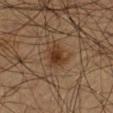Clinical impression:
The lesion was photographed on a routine skin check and not biopsied; there is no pathology result.
Context:
A male subject, in their mid-60s. On the left thigh. Cropped from a whole-body photographic skin survey; the tile spans about 15 mm. An algorithmic analysis of the crop reported about 8 CIELAB-L* units darker than the surrounding skin and a lesion-to-skin contrast of about 9 (normalized; higher = more distinct). It also reported border irregularity of about 3 on a 0–10 scale and a peripheral color-asymmetry measure near 1. And it measured a classifier nevus-likeness of about 90/100 and a detector confidence of about 100 out of 100 that the crop contains a lesion. Captured under cross-polarized illumination.